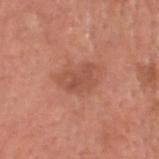Q: Was a biopsy performed?
A: imaged on a skin check; not biopsied
Q: What is the imaging modality?
A: ~15 mm crop, total-body skin-cancer survey
Q: Patient demographics?
A: male, about 65 years old
Q: Lesion size?
A: ≈4 mm
Q: Automated lesion metrics?
A: a shape eccentricity near 0.75 and a symmetry-axis asymmetry near 0.4
Q: Lesion location?
A: the head or neck
Q: Illumination type?
A: white-light illumination